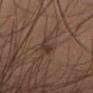• follow-up — no biopsy performed (imaged during a skin exam)
• automated lesion analysis — a border-irregularity index near 4.5/10 and a color-variation rating of about 1.5/10
• diameter — ≈4 mm
• subject — male, approximately 35 years of age
• location — the right thigh
• image source — 15 mm crop, total-body photography